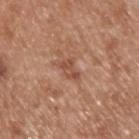The patient is a male aged approximately 65.
About 2.5 mm across.
Located on the upper back.
A close-up tile cropped from a whole-body skin photograph, about 15 mm across.